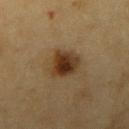This lesion was catalogued during total-body skin photography and was not selected for biopsy.
A 15 mm crop from a total-body photograph taken for skin-cancer surveillance.
This is a cross-polarized tile.
Longest diameter approximately 4 mm.
The patient is a male aged approximately 60.
Located on the right upper arm.